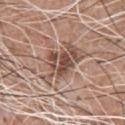{
  "biopsy_status": "not biopsied; imaged during a skin examination",
  "lesion_size": {
    "long_diameter_mm_approx": 6.0
  },
  "patient": {
    "sex": "male",
    "age_approx": 60
  },
  "automated_metrics": {
    "area_mm2_approx": 16.0,
    "eccentricity": 0.8,
    "shape_asymmetry": 0.4,
    "cielab_L": 51,
    "cielab_a": 18,
    "cielab_b": 25,
    "vs_skin_darker_L": 11.0,
    "vs_skin_contrast_norm": 8.0,
    "border_irregularity_0_10": 6.5,
    "color_variation_0_10": 6.5,
    "peripheral_color_asymmetry": 2.0
  },
  "site": "chest",
  "image": {
    "source": "total-body photography crop",
    "field_of_view_mm": 15
  }
}